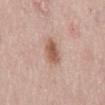Notes:
• biopsy status — no biopsy performed (imaged during a skin exam)
• image — 15 mm crop, total-body photography
• lighting — white-light
• image-analysis metrics — an area of roughly 6 mm², an outline eccentricity of about 0.7 (0 = round, 1 = elongated), and two-axis asymmetry of about 0.2; a lesion color around L≈57 a*≈21 b*≈28 in CIELAB and roughly 12 lightness units darker than nearby skin; a border-irregularity index near 2/10, a within-lesion color-variation index near 3.5/10, and radial color variation of about 1; a classifier nevus-likeness of about 95/100 and a lesion-detection confidence of about 100/100
• diameter — ≈3 mm
• subject — male, about 80 years old
• location — the back